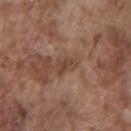From the chest. The tile uses white-light illumination. A male patient, in their mid-70s. A 15 mm close-up extracted from a 3D total-body photography capture. Automated tile analysis of the lesion measured an area of roughly 3.5 mm², an eccentricity of roughly 0.85, and two-axis asymmetry of about 0.35. And it measured a lesion color around L≈43 a*≈19 b*≈27 in CIELAB, about 8 CIELAB-L* units darker than the surrounding skin, and a normalized lesion–skin contrast near 7. The software also gave a border-irregularity rating of about 4/10 and peripheral color asymmetry of about 0.5. And it measured a lesion-detection confidence of about 80/100. The lesion's longest dimension is about 3 mm.